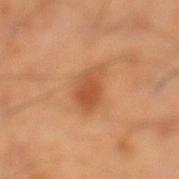Assessment:
The lesion was photographed on a routine skin check and not biopsied; there is no pathology result.
Clinical summary:
A male patient aged 58–62. Cropped from a whole-body photographic skin survey; the tile spans about 15 mm. The lesion's longest dimension is about 4 mm. The lesion-visualizer software estimated a color-variation rating of about 3.5/10 and a peripheral color-asymmetry measure near 1. The lesion is located on the left lower leg. This is a cross-polarized tile.